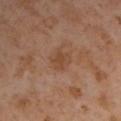No biopsy was performed on this lesion — it was imaged during a full skin examination and was not determined to be concerning. Longest diameter approximately 3 mm. The subject is a male in their mid- to late 50s. A 15 mm crop from a total-body photograph taken for skin-cancer surveillance. Imaged with cross-polarized lighting. The lesion is on the left upper arm.